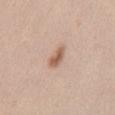Automated tile analysis of the lesion measured an area of roughly 4 mm², an eccentricity of roughly 0.85, and a shape-asymmetry score of about 0.35 (0 = symmetric). And it measured internal color variation of about 2 on a 0–10 scale and a peripheral color-asymmetry measure near 0.5. The analysis additionally found a classifier nevus-likeness of about 85/100 and a lesion-detection confidence of about 100/100. Cropped from a total-body skin-imaging series; the visible field is about 15 mm. Imaged with white-light lighting. A male patient, approximately 45 years of age. On the abdomen.